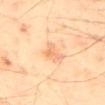{
  "biopsy_status": "not biopsied; imaged during a skin examination",
  "lesion_size": {
    "long_diameter_mm_approx": 3.0
  },
  "image": {
    "source": "total-body photography crop",
    "field_of_view_mm": 15
  },
  "site": "mid back",
  "patient": {
    "sex": "male",
    "age_approx": 40
  },
  "lighting": "cross-polarized"
}